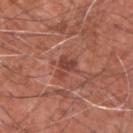biopsy status = no biopsy performed (imaged during a skin exam); image = total-body-photography crop, ~15 mm field of view; lesion size = about 2.5 mm; site = the upper back; tile lighting = white-light; patient = male, in their 60s.